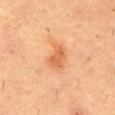Case summary:
* image source · total-body-photography crop, ~15 mm field of view
* lighting · cross-polarized
* subject · male, aged around 55
* location · the mid back